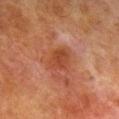Q: Was a biopsy performed?
A: imaged on a skin check; not biopsied
Q: What is the anatomic site?
A: the right lower leg
Q: What is the lesion's diameter?
A: about 3.5 mm
Q: How was the tile lit?
A: cross-polarized
Q: What kind of image is this?
A: 15 mm crop, total-body photography
Q: Patient demographics?
A: male, approximately 80 years of age